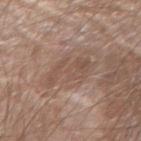  biopsy_status: not biopsied; imaged during a skin examination
  lesion_size:
    long_diameter_mm_approx: 5.5
  lighting: white-light
  image:
    source: total-body photography crop
    field_of_view_mm: 15
  patient:
    sex: male
    age_approx: 60
  site: left forearm